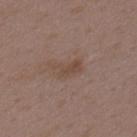Q: Was a biopsy performed?
A: imaged on a skin check; not biopsied
Q: Patient demographics?
A: female, aged approximately 35
Q: What kind of image is this?
A: ~15 mm crop, total-body skin-cancer survey
Q: Lesion location?
A: the upper back